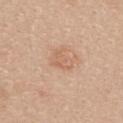Case summary:
• notes · total-body-photography surveillance lesion; no biopsy
• patient · female, aged 23–27
• body site · the upper back
• lesion size · ~3 mm (longest diameter)
• automated lesion analysis · a lesion area of about 5 mm², a shape eccentricity near 0.75, and a shape-asymmetry score of about 0.25 (0 = symmetric); a border-irregularity rating of about 2.5/10 and a color-variation rating of about 2.5/10; a classifier nevus-likeness of about 0/100
• acquisition · 15 mm crop, total-body photography